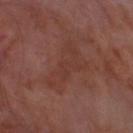Findings:
- workup · no biopsy performed (imaged during a skin exam)
- diameter · ≈7 mm
- acquisition · total-body-photography crop, ~15 mm field of view
- tile lighting · cross-polarized
- subject · male, roughly 70 years of age
- site · the left lower leg